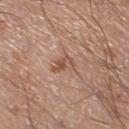{
  "biopsy_status": "not biopsied; imaged during a skin examination",
  "site": "left lower leg",
  "automated_metrics": {
    "area_mm2_approx": 4.0,
    "eccentricity": 0.8,
    "shape_asymmetry": 0.4,
    "cielab_L": 53,
    "cielab_a": 21,
    "cielab_b": 28,
    "vs_skin_darker_L": 9.0,
    "vs_skin_contrast_norm": 6.5,
    "border_irregularity_0_10": 4.0,
    "color_variation_0_10": 5.0,
    "peripheral_color_asymmetry": 1.5
  },
  "image": {
    "source": "total-body photography crop",
    "field_of_view_mm": 15
  },
  "lesion_size": {
    "long_diameter_mm_approx": 2.5
  },
  "patient": {
    "sex": "male",
    "age_approx": 20
  },
  "lighting": "white-light"
}